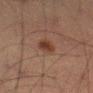notes=total-body-photography surveillance lesion; no biopsy | image-analysis metrics=a lesion color around L≈30 a*≈17 b*≈23 in CIELAB and about 8 CIELAB-L* units darker than the surrounding skin; a nevus-likeness score of about 90/100 and a detector confidence of about 100 out of 100 that the crop contains a lesion | lesion diameter=~3 mm (longest diameter) | patient=male, about 65 years old | site=the leg | tile lighting=cross-polarized | image=15 mm crop, total-body photography.